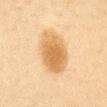| field | value |
|---|---|
| notes | imaged on a skin check; not biopsied |
| subject | female, aged 38 to 42 |
| location | the chest |
| image | total-body-photography crop, ~15 mm field of view |
| diameter | about 5.5 mm |
| automated metrics | a lesion color around L≈58 a*≈17 b*≈39 in CIELAB, roughly 11 lightness units darker than nearby skin, and a lesion-to-skin contrast of about 8 (normalized; higher = more distinct) |
| tile lighting | cross-polarized illumination |